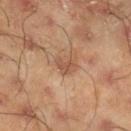Assessment: Recorded during total-body skin imaging; not selected for excision or biopsy. Image and clinical context: About 2.5 mm across. A close-up tile cropped from a whole-body skin photograph, about 15 mm across. The lesion is on the right lower leg. A male subject, aged around 45. Automated tile analysis of the lesion measured a border-irregularity rating of about 4/10, a color-variation rating of about 1.5/10, and peripheral color asymmetry of about 0.5. It also reported a lesion-detection confidence of about 100/100. The tile uses cross-polarized illumination.